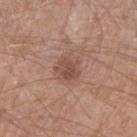Impression:
Part of a total-body skin-imaging series; this lesion was reviewed on a skin check and was not flagged for biopsy.
Background:
The lesion's longest dimension is about 3.5 mm. The lesion is located on the arm. Imaged with white-light lighting. Cropped from a whole-body photographic skin survey; the tile spans about 15 mm. A male subject, aged approximately 55.